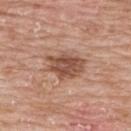Impression:
The lesion was tiled from a total-body skin photograph and was not biopsied.
Acquisition and patient details:
From the chest. The tile uses white-light illumination. The subject is a female in their mid- to late 60s. A 15 mm close-up extracted from a 3D total-body photography capture.